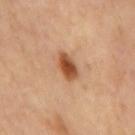This lesion was catalogued during total-body skin photography and was not selected for biopsy. The lesion's longest dimension is about 4 mm. The lesion is on the back. A roughly 15 mm field-of-view crop from a total-body skin photograph. The lesion-visualizer software estimated a footprint of about 6 mm², a shape eccentricity near 0.85, and a shape-asymmetry score of about 0.15 (0 = symmetric). And it measured a border-irregularity index near 2/10, a color-variation rating of about 4.5/10, and peripheral color asymmetry of about 1. And it measured an automated nevus-likeness rating near 100 out of 100 and lesion-presence confidence of about 100/100. The tile uses cross-polarized illumination.